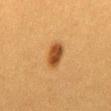<case>
<biopsy_status>not biopsied; imaged during a skin examination</biopsy_status>
<lighting>cross-polarized</lighting>
<image>
  <source>total-body photography crop</source>
  <field_of_view_mm>15</field_of_view_mm>
</image>
<patient>
  <sex>female</sex>
  <age_approx>40</age_approx>
</patient>
<lesion_size>
  <long_diameter_mm_approx>3.5</long_diameter_mm_approx>
</lesion_size>
<automated_metrics>
  <area_mm2_approx>6.5</area_mm2_approx>
  <shape_asymmetry>0.2</shape_asymmetry>
  <cielab_L>41</cielab_L>
  <cielab_a>21</cielab_a>
  <cielab_b>36</cielab_b>
  <vs_skin_darker_L>13.0</vs_skin_darker_L>
  <vs_skin_contrast_norm>10.0</vs_skin_contrast_norm>
  <border_irregularity_0_10>2.0</border_irregularity_0_10>
  <color_variation_0_10>3.0</color_variation_0_10>
  <peripheral_color_asymmetry>1.0</peripheral_color_asymmetry>
  <lesion_detection_confidence_0_100>100</lesion_detection_confidence_0_100>
</automated_metrics>
<site>mid back</site>
</case>